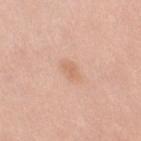biopsy_status: not biopsied; imaged during a skin examination
patient:
  sex: female
  age_approx: 65
site: right upper arm
image:
  source: total-body photography crop
  field_of_view_mm: 15